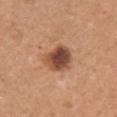Part of a total-body skin-imaging series; this lesion was reviewed on a skin check and was not flagged for biopsy.
The recorded lesion diameter is about 3.5 mm.
Cropped from a total-body skin-imaging series; the visible field is about 15 mm.
The total-body-photography lesion software estimated an area of roughly 10 mm² and an eccentricity of roughly 0.5. And it measured a mean CIELAB color near L≈48 a*≈24 b*≈31, about 16 CIELAB-L* units darker than the surrounding skin, and a normalized border contrast of about 11. It also reported border irregularity of about 2 on a 0–10 scale, internal color variation of about 6.5 on a 0–10 scale, and a peripheral color-asymmetry measure near 1.5.
From the right upper arm.
A female subject, in their mid- to late 50s.